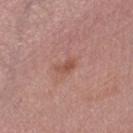Assessment:
This lesion was catalogued during total-body skin photography and was not selected for biopsy.
Clinical summary:
Located on the leg. A female patient approximately 65 years of age. The lesion-visualizer software estimated a footprint of about 3 mm² and a shape eccentricity near 0.85. The software also gave an average lesion color of about L≈51 a*≈23 b*≈28 (CIELAB), about 8 CIELAB-L* units darker than the surrounding skin, and a normalized lesion–skin contrast near 6.5. And it measured a border-irregularity index near 4/10 and a peripheral color-asymmetry measure near 0. And it measured lesion-presence confidence of about 100/100. This is a white-light tile. A 15 mm crop from a total-body photograph taken for skin-cancer surveillance. Measured at roughly 2.5 mm in maximum diameter.